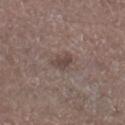Context: Automated image analysis of the tile measured an area of roughly 4.5 mm² and an eccentricity of roughly 0.6. The analysis additionally found an automated nevus-likeness rating near 0 out of 100. A close-up tile cropped from a whole-body skin photograph, about 15 mm across. The subject is a male in their mid-60s. This is a white-light tile. Longest diameter approximately 2.5 mm. From the right lower leg.